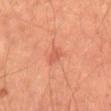Q: Was a biopsy performed?
A: catalogued during a skin exam; not biopsied
Q: Where on the body is the lesion?
A: the abdomen
Q: What lighting was used for the tile?
A: cross-polarized
Q: What kind of image is this?
A: 15 mm crop, total-body photography
Q: How large is the lesion?
A: about 2.5 mm
Q: Patient demographics?
A: male, aged around 65